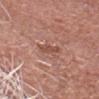{
  "biopsy_status": "not biopsied; imaged during a skin examination",
  "lighting": "white-light",
  "image": {
    "source": "total-body photography crop",
    "field_of_view_mm": 15
  },
  "site": "head or neck",
  "patient": {
    "sex": "male",
    "age_approx": 65
  },
  "lesion_size": {
    "long_diameter_mm_approx": 2.5
  },
  "automated_metrics": {
    "area_mm2_approx": 3.5,
    "eccentricity": 0.8,
    "shape_asymmetry": 0.3,
    "cielab_L": 50,
    "cielab_a": 24,
    "cielab_b": 28,
    "vs_skin_darker_L": 8.0,
    "vs_skin_contrast_norm": 6.5,
    "nevus_likeness_0_100": 0
  }
}